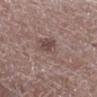Recorded during total-body skin imaging; not selected for excision or biopsy. A 15 mm close-up extracted from a 3D total-body photography capture. The lesion is on the leg. Approximately 4.5 mm at its widest. This is a white-light tile. A male subject aged 68 to 72.